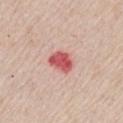Part of a total-body skin-imaging series; this lesion was reviewed on a skin check and was not flagged for biopsy. A female patient aged approximately 45. Automated image analysis of the tile measured about 16 CIELAB-L* units darker than the surrounding skin. A lesion tile, about 15 mm wide, cut from a 3D total-body photograph. From the chest. The recorded lesion diameter is about 3 mm.